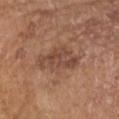Impression: Imaged during a routine full-body skin examination; the lesion was not biopsied and no histopathology is available. Acquisition and patient details: A female subject aged approximately 70. From the right forearm. This image is a 15 mm lesion crop taken from a total-body photograph.